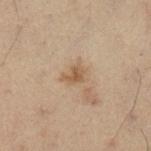The lesion's longest dimension is about 2.5 mm. The subject is a male aged 53–57. A 15 mm close-up tile from a total-body photography series done for melanoma screening. The total-body-photography lesion software estimated a mean CIELAB color near L≈45 a*≈13 b*≈27, about 8 CIELAB-L* units darker than the surrounding skin, and a normalized lesion–skin contrast near 7. It also reported a classifier nevus-likeness of about 10/100 and a lesion-detection confidence of about 100/100. Imaged with cross-polarized lighting. The lesion is on the leg.